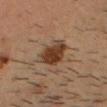The lesion was tiled from a total-body skin photograph and was not biopsied. The lesion-visualizer software estimated a mean CIELAB color near L≈34 a*≈17 b*≈28 and a normalized border contrast of about 11. The software also gave a border-irregularity rating of about 2.5/10, internal color variation of about 4 on a 0–10 scale, and peripheral color asymmetry of about 1.5. A close-up tile cropped from a whole-body skin photograph, about 15 mm across. The lesion is on the head or neck. Imaged with cross-polarized lighting. A male patient roughly 35 years of age. The recorded lesion diameter is about 4 mm.